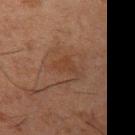notes: no biopsy performed (imaged during a skin exam); lesion size: ≈3.5 mm; body site: the arm; image source: ~15 mm tile from a whole-body skin photo; subject: male, aged around 50.